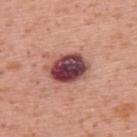Q: Is there a histopathology result?
A: imaged on a skin check; not biopsied
Q: Lesion location?
A: the upper back
Q: How large is the lesion?
A: ~5 mm (longest diameter)
Q: Who is the patient?
A: male, aged 68–72
Q: What is the imaging modality?
A: 15 mm crop, total-body photography
Q: How was the tile lit?
A: white-light illumination